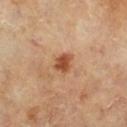Q: Was this lesion biopsied?
A: imaged on a skin check; not biopsied
Q: What is the lesion's diameter?
A: ≈2.5 mm
Q: What lighting was used for the tile?
A: cross-polarized illumination
Q: What is the imaging modality?
A: ~15 mm tile from a whole-body skin photo
Q: What are the patient's age and sex?
A: male, aged 63 to 67
Q: Lesion location?
A: the right lower leg